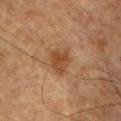Assessment:
This lesion was catalogued during total-body skin photography and was not selected for biopsy.
Background:
A region of skin cropped from a whole-body photographic capture, roughly 15 mm wide. Measured at roughly 3.5 mm in maximum diameter. On the front of the torso. A male patient in their 50s. Automated image analysis of the tile measured a mean CIELAB color near L≈37 a*≈18 b*≈29, a lesion–skin lightness drop of about 8, and a lesion-to-skin contrast of about 7.5 (normalized; higher = more distinct). It also reported a border-irregularity index near 3/10, internal color variation of about 2.5 on a 0–10 scale, and peripheral color asymmetry of about 1. Imaged with cross-polarized lighting.